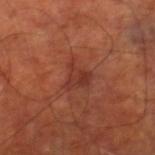The lesion was photographed on a routine skin check and not biopsied; there is no pathology result. This is a cross-polarized tile. A patient aged 63–67. From the right lower leg. A close-up tile cropped from a whole-body skin photograph, about 15 mm across.